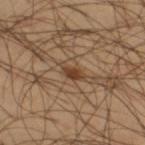The lesion was tiled from a total-body skin photograph and was not biopsied. The lesion is located on the right thigh. The patient is a male aged 43–47. The recorded lesion diameter is about 3 mm. A lesion tile, about 15 mm wide, cut from a 3D total-body photograph. The lesion-visualizer software estimated a footprint of about 3.5 mm² and two-axis asymmetry of about 0.3. The software also gave a lesion color around L≈40 a*≈17 b*≈30 in CIELAB. And it measured border irregularity of about 3.5 on a 0–10 scale, internal color variation of about 1.5 on a 0–10 scale, and peripheral color asymmetry of about 0.5. The analysis additionally found an automated nevus-likeness rating near 75 out of 100 and a lesion-detection confidence of about 100/100. Captured under cross-polarized illumination.